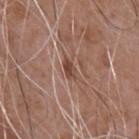Q: Is there a histopathology result?
A: no biopsy performed (imaged during a skin exam)
Q: What are the patient's age and sex?
A: male, approximately 75 years of age
Q: Lesion location?
A: the chest
Q: Lesion size?
A: ≈2.5 mm
Q: What is the imaging modality?
A: ~15 mm tile from a whole-body skin photo
Q: How was the tile lit?
A: white-light